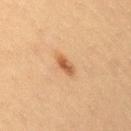Captured during whole-body skin photography for melanoma surveillance; the lesion was not biopsied.
From the right thigh.
This is a cross-polarized tile.
Longest diameter approximately 2.5 mm.
A lesion tile, about 15 mm wide, cut from a 3D total-body photograph.
A female patient, approximately 40 years of age.